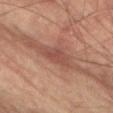Clinical impression:
Part of a total-body skin-imaging series; this lesion was reviewed on a skin check and was not flagged for biopsy.
Background:
A male patient, approximately 65 years of age. The tile uses cross-polarized illumination. A close-up tile cropped from a whole-body skin photograph, about 15 mm across. From the left thigh. The lesion-visualizer software estimated an eccentricity of roughly 0.9 and a shape-asymmetry score of about 0.3 (0 = symmetric). The software also gave a lesion color around L≈43 a*≈21 b*≈24 in CIELAB, about 8 CIELAB-L* units darker than the surrounding skin, and a normalized lesion–skin contrast near 6.5. The software also gave lesion-presence confidence of about 60/100. About 4 mm across.